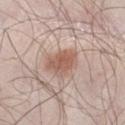The lesion was tiled from a total-body skin photograph and was not biopsied.
A male subject, in their mid-50s.
On the right thigh.
A roughly 15 mm field-of-view crop from a total-body skin photograph.